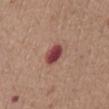biopsy_status: not biopsied; imaged during a skin examination
site: front of the torso
patient:
  sex: female
  age_approx: 75
image:
  source: total-body photography crop
  field_of_view_mm: 15